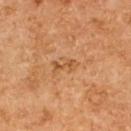| feature | finding |
|---|---|
| workup | catalogued during a skin exam; not biopsied |